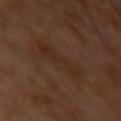workup=no biopsy performed (imaged during a skin exam) | patient=male, aged 28 to 32 | imaging modality=total-body-photography crop, ~15 mm field of view | site=the left upper arm | TBP lesion metrics=roughly 4 lightness units darker than nearby skin and a normalized border contrast of about 5; an automated nevus-likeness rating near 0 out of 100 and a detector confidence of about 100 out of 100 that the crop contains a lesion | lighting=cross-polarized illumination.